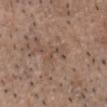Findings:
* workup: no biopsy performed (imaged during a skin exam)
* patient: male, approximately 50 years of age
* image: 15 mm crop, total-body photography
* automated lesion analysis: an average lesion color of about L≈49 a*≈16 b*≈26 (CIELAB), about 6 CIELAB-L* units darker than the surrounding skin, and a normalized lesion–skin contrast near 4.5; a border-irregularity rating of about 5/10 and a within-lesion color-variation index near 0/10; a classifier nevus-likeness of about 0/100
* site: the head or neck